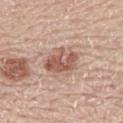Impression: The lesion was photographed on a routine skin check and not biopsied; there is no pathology result. Acquisition and patient details: The total-body-photography lesion software estimated a shape eccentricity near 0.7 and a shape-asymmetry score of about 0.3 (0 = symmetric). It also reported border irregularity of about 3.5 on a 0–10 scale, a within-lesion color-variation index near 4.5/10, and radial color variation of about 1.5. A 15 mm crop from a total-body photograph taken for skin-cancer surveillance. About 4 mm across. The tile uses white-light illumination. From the mid back. The patient is a male in their 60s.